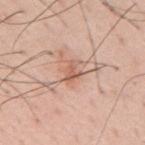Image and clinical context:
A lesion tile, about 15 mm wide, cut from a 3D total-body photograph. A male subject, approximately 55 years of age. The lesion is on the mid back.